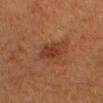  biopsy_status: not biopsied; imaged during a skin examination
  image:
    source: total-body photography crop
    field_of_view_mm: 15
  lighting: cross-polarized
  patient:
    sex: female
    age_approx: 40
  site: head or neck